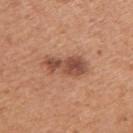Findings:
* biopsy status · total-body-photography surveillance lesion; no biopsy
* anatomic site · the right upper arm
* acquisition · 15 mm crop, total-body photography
* automated metrics · a footprint of about 12 mm², an eccentricity of roughly 0.9, and a symmetry-axis asymmetry near 0.3; a lesion color around L≈51 a*≈23 b*≈30 in CIELAB, a lesion–skin lightness drop of about 12, and a normalized lesion–skin contrast near 8
* diameter · ~5.5 mm (longest diameter)
* subject · male, aged 53 to 57
* tile lighting · white-light illumination